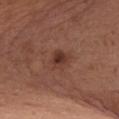Impression:
Recorded during total-body skin imaging; not selected for excision or biopsy.
Image and clinical context:
Cropped from a total-body skin-imaging series; the visible field is about 15 mm. Captured under white-light illumination. Longest diameter approximately 3 mm. A female patient, aged 53 to 57. The lesion is on the chest.